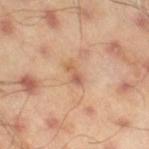workup: total-body-photography surveillance lesion; no biopsy
patient: male, aged 43–47
automated lesion analysis: a lesion area of about 2.5 mm² and a shape-asymmetry score of about 0.4 (0 = symmetric); an average lesion color of about L≈58 a*≈22 b*≈33 (CIELAB) and a lesion-to-skin contrast of about 6 (normalized; higher = more distinct)
diameter: about 2.5 mm
acquisition: ~15 mm tile from a whole-body skin photo
location: the right thigh
lighting: cross-polarized